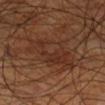Findings:
- biopsy status: total-body-photography surveillance lesion; no biopsy
- site: the right lower leg
- TBP lesion metrics: an average lesion color of about L≈30 a*≈19 b*≈26 (CIELAB), roughly 5 lightness units darker than nearby skin, and a normalized lesion–skin contrast near 5.5; internal color variation of about 4.5 on a 0–10 scale and a peripheral color-asymmetry measure near 1.5; a nevus-likeness score of about 0/100 and lesion-presence confidence of about 80/100
- patient: male, aged 68 to 72
- acquisition: total-body-photography crop, ~15 mm field of view
- size: about 8.5 mm
- lighting: cross-polarized illumination